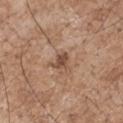Acquisition and patient details:
A close-up tile cropped from a whole-body skin photograph, about 15 mm across. Longest diameter approximately 2.5 mm. From the right upper arm. The patient is a male about 70 years old. The total-body-photography lesion software estimated a mean CIELAB color near L≈48 a*≈19 b*≈29, about 11 CIELAB-L* units darker than the surrounding skin, and a lesion-to-skin contrast of about 8 (normalized; higher = more distinct). The software also gave border irregularity of about 3.5 on a 0–10 scale, a within-lesion color-variation index near 1.5/10, and radial color variation of about 0.5. The analysis additionally found lesion-presence confidence of about 100/100.